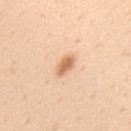Impression: No biopsy was performed on this lesion — it was imaged during a full skin examination and was not determined to be concerning. Background: Located on the upper back. Captured under white-light illumination. A close-up tile cropped from a whole-body skin photograph, about 15 mm across. A male subject aged 58 to 62.